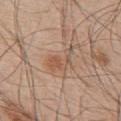Impression: The lesion was tiled from a total-body skin photograph and was not biopsied. Image and clinical context: A male patient, aged approximately 55. The lesion-visualizer software estimated a color-variation rating of about 2/10 and radial color variation of about 0.5. It also reported a nevus-likeness score of about 15/100 and a lesion-detection confidence of about 100/100. The lesion is on the upper back. Cropped from a whole-body photographic skin survey; the tile spans about 15 mm.